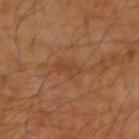Q: Was a biopsy performed?
A: catalogued during a skin exam; not biopsied
Q: Illumination type?
A: cross-polarized
Q: How was this image acquired?
A: ~15 mm crop, total-body skin-cancer survey
Q: Where on the body is the lesion?
A: the arm
Q: Patient demographics?
A: male, roughly 60 years of age
Q: How large is the lesion?
A: ≈3.5 mm